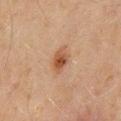Assessment: Captured during whole-body skin photography for melanoma surveillance; the lesion was not biopsied. Acquisition and patient details: Captured under cross-polarized illumination. Automated tile analysis of the lesion measured a footprint of about 5 mm², a shape eccentricity near 0.75, and a symmetry-axis asymmetry near 0.2. The software also gave border irregularity of about 2 on a 0–10 scale and peripheral color asymmetry of about 1. It also reported a nevus-likeness score of about 95/100 and a detector confidence of about 100 out of 100 that the crop contains a lesion. A roughly 15 mm field-of-view crop from a total-body skin photograph. From the mid back. A male subject, aged 43 to 47. The recorded lesion diameter is about 3 mm.